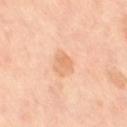Imaged during a routine full-body skin examination; the lesion was not biopsied and no histopathology is available. The patient is a female in their 50s. A 15 mm close-up extracted from a 3D total-body photography capture. Captured under cross-polarized illumination. From the right thigh. The recorded lesion diameter is about 3 mm.